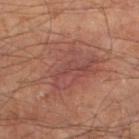{"automated_metrics": {"area_mm2_approx": 30.0, "eccentricity": 0.65, "shape_asymmetry": 0.4, "cielab_L": 46, "cielab_a": 23, "cielab_b": 25, "vs_skin_darker_L": 7.0, "vs_skin_contrast_norm": 6.0, "border_irregularity_0_10": 7.0, "color_variation_0_10": 4.5}, "lesion_size": {"long_diameter_mm_approx": 8.5}, "image": {"source": "total-body photography crop", "field_of_view_mm": 15}, "site": "left lower leg", "patient": {"sex": "male", "age_approx": 65}}